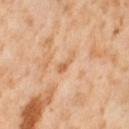The lesion was tiled from a total-body skin photograph and was not biopsied. The patient is a female aged around 55. Captured under cross-polarized illumination. The lesion's longest dimension is about 3 mm. A roughly 15 mm field-of-view crop from a total-body skin photograph. The lesion is on the right thigh.